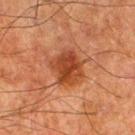Assessment:
Recorded during total-body skin imaging; not selected for excision or biopsy.
Acquisition and patient details:
This image is a 15 mm lesion crop taken from a total-body photograph. About 4 mm across. Captured under cross-polarized illumination. The total-body-photography lesion software estimated an average lesion color of about L≈35 a*≈24 b*≈31 (CIELAB), about 10 CIELAB-L* units darker than the surrounding skin, and a normalized border contrast of about 8.5. And it measured a classifier nevus-likeness of about 85/100 and a lesion-detection confidence of about 100/100. The lesion is on the leg. The patient is a male aged 78–82.